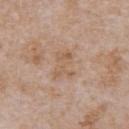Clinical impression: The lesion was photographed on a routine skin check and not biopsied; there is no pathology result. Context: A lesion tile, about 15 mm wide, cut from a 3D total-body photograph. Measured at roughly 3.5 mm in maximum diameter. The patient is a male aged 63 to 67. Located on the chest.